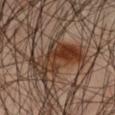– biopsy status: catalogued during a skin exam; not biopsied
– acquisition: total-body-photography crop, ~15 mm field of view
– site: the left thigh
– size: ≈8 mm
– patient: male, aged 43 to 47
– tile lighting: cross-polarized